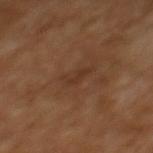Part of a total-body skin-imaging series; this lesion was reviewed on a skin check and was not flagged for biopsy.
A 15 mm crop from a total-body photograph taken for skin-cancer surveillance.
The subject is a male aged 63 to 67.